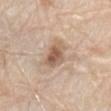<record>
<patient>
  <sex>male</sex>
  <age_approx>80</age_approx>
</patient>
<automated_metrics>
  <nevus_likeness_0_100>85</nevus_likeness_0_100>
  <lesion_detection_confidence_0_100>100</lesion_detection_confidence_0_100>
</automated_metrics>
<image>
  <source>total-body photography crop</source>
  <field_of_view_mm>15</field_of_view_mm>
</image>
<site>abdomen</site>
<lighting>white-light</lighting>
</record>